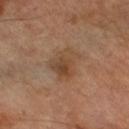Part of a total-body skin-imaging series; this lesion was reviewed on a skin check and was not flagged for biopsy. A 15 mm crop from a total-body photograph taken for skin-cancer surveillance. Measured at roughly 4.5 mm in maximum diameter. Captured under cross-polarized illumination. Automated image analysis of the tile measured a nevus-likeness score of about 0/100. A male patient, aged 68–72. The lesion is located on the right thigh.